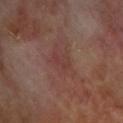Notes:
• follow-up · no biopsy performed (imaged during a skin exam)
• site · the upper back
• automated lesion analysis · an average lesion color of about L≈36 a*≈22 b*≈22 (CIELAB) and a lesion–skin lightness drop of about 5
• lesion diameter · about 3 mm
• imaging modality · 15 mm crop, total-body photography
• patient · male, aged approximately 70